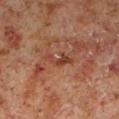workup = total-body-photography surveillance lesion; no biopsy
body site = the left lower leg
subject = male, roughly 60 years of age
image source = ~15 mm tile from a whole-body skin photo